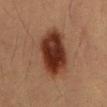Clinical impression:
No biopsy was performed on this lesion — it was imaged during a full skin examination and was not determined to be concerning.
Background:
An algorithmic analysis of the crop reported a shape-asymmetry score of about 0.15 (0 = symmetric). And it measured a lesion-detection confidence of about 100/100. A 15 mm close-up tile from a total-body photography series done for melanoma screening. A male subject, roughly 55 years of age. On the abdomen. Imaged with cross-polarized lighting. Measured at roughly 7 mm in maximum diameter.